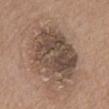workup: total-body-photography surveillance lesion; no biopsy | automated lesion analysis: a mean CIELAB color near L≈47 a*≈14 b*≈25 and a lesion–skin lightness drop of about 12; border irregularity of about 2.5 on a 0–10 scale and a peripheral color-asymmetry measure near 2.5; an automated nevus-likeness rating near 5 out of 100 | anatomic site: the abdomen | tile lighting: white-light | imaging modality: ~15 mm crop, total-body skin-cancer survey | subject: male, roughly 75 years of age.